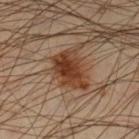<lesion>
<patient>
  <sex>male</sex>
  <age_approx>45</age_approx>
</patient>
<image>
  <source>total-body photography crop</source>
  <field_of_view_mm>15</field_of_view_mm>
</image>
<lighting>cross-polarized</lighting>
<site>left lower leg</site>
<lesion_size>
  <long_diameter_mm_approx>5.0</long_diameter_mm_approx>
</lesion_size>
</lesion>